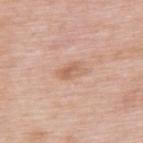follow-up: imaged on a skin check; not biopsied | patient: male, roughly 55 years of age | imaging modality: total-body-photography crop, ~15 mm field of view | anatomic site: the upper back.